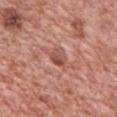Part of a total-body skin-imaging series; this lesion was reviewed on a skin check and was not flagged for biopsy. The subject is a male aged 48 to 52. The lesion is located on the front of the torso. A lesion tile, about 15 mm wide, cut from a 3D total-body photograph.